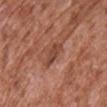notes: total-body-photography surveillance lesion; no biopsy | automated lesion analysis: a footprint of about 4.5 mm², a shape eccentricity near 0.9, and a symmetry-axis asymmetry near 0.3 | location: the chest | illumination: white-light | patient: male, aged around 75 | image: 15 mm crop, total-body photography.